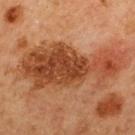Assessment:
The lesion was photographed on a routine skin check and not biopsied; there is no pathology result.
Background:
From the mid back. Cropped from a whole-body photographic skin survey; the tile spans about 15 mm. A male patient, aged 63 to 67.